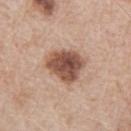Q: Is there a histopathology result?
A: total-body-photography surveillance lesion; no biopsy
Q: What is the lesion's diameter?
A: ~4.5 mm (longest diameter)
Q: How was this image acquired?
A: 15 mm crop, total-body photography
Q: What did automated image analysis measure?
A: an area of roughly 13 mm² and two-axis asymmetry of about 0.3
Q: Lesion location?
A: the arm
Q: What are the patient's age and sex?
A: male, in their mid-60s